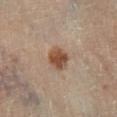Q: Who is the patient?
A: female, approximately 60 years of age
Q: How large is the lesion?
A: ≈3 mm
Q: Where on the body is the lesion?
A: the leg
Q: What is the imaging modality?
A: ~15 mm tile from a whole-body skin photo
Q: What lighting was used for the tile?
A: cross-polarized